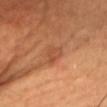workup=total-body-photography surveillance lesion; no biopsy | lesion size=about 2.5 mm | imaging modality=~15 mm crop, total-body skin-cancer survey | anatomic site=the chest | patient=male, in their mid-60s.